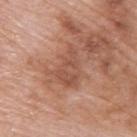workup: catalogued during a skin exam; not biopsied
image source: ~15 mm crop, total-body skin-cancer survey
diameter: ~4.5 mm (longest diameter)
location: the upper back
tile lighting: white-light
TBP lesion metrics: a footprint of about 12 mm² and a shape-asymmetry score of about 0.4 (0 = symmetric)
patient: male, roughly 80 years of age